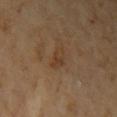{
  "patient": {
    "sex": "female",
    "age_approx": 60
  },
  "image": {
    "source": "total-body photography crop",
    "field_of_view_mm": 15
  },
  "site": "left upper arm"
}